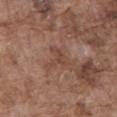Impression: Recorded during total-body skin imaging; not selected for excision or biopsy. Acquisition and patient details: A male patient, aged 73 to 77. A 15 mm close-up extracted from a 3D total-body photography capture. Captured under white-light illumination. Measured at roughly 2.5 mm in maximum diameter. Located on the abdomen. An algorithmic analysis of the crop reported an average lesion color of about L≈45 a*≈20 b*≈26 (CIELAB), roughly 6 lightness units darker than nearby skin, and a normalized lesion–skin contrast near 5. It also reported an automated nevus-likeness rating near 0 out of 100 and a lesion-detection confidence of about 95/100.